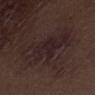{
  "biopsy_status": "not biopsied; imaged during a skin examination",
  "lighting": "white-light",
  "site": "right thigh",
  "patient": {
    "sex": "male",
    "age_approx": 70
  },
  "image": {
    "source": "total-body photography crop",
    "field_of_view_mm": 15
  }
}